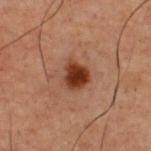An algorithmic analysis of the crop reported a lesion area of about 7 mm². The recorded lesion diameter is about 3.5 mm. This is a cross-polarized tile. Located on the front of the torso. Cropped from a total-body skin-imaging series; the visible field is about 15 mm. A male subject, aged 58 to 62.